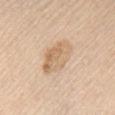Image and clinical context: The patient is a male aged 68–72. Measured at roughly 5 mm in maximum diameter. Automated image analysis of the tile measured an outline eccentricity of about 0.8 (0 = round, 1 = elongated). And it measured a mean CIELAB color near L≈67 a*≈16 b*≈34 and a normalized border contrast of about 6. It also reported a color-variation rating of about 5/10. A 15 mm crop from a total-body photograph taken for skin-cancer surveillance. Imaged with white-light lighting. On the front of the torso.